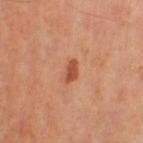image source: total-body-photography crop, ~15 mm field of view
site: the leg
subject: male, approximately 65 years of age
illumination: cross-polarized